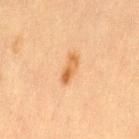- follow-up: no biopsy performed (imaged during a skin exam)
- image source: 15 mm crop, total-body photography
- site: the left thigh
- size: ~3.5 mm (longest diameter)
- patient: female, in their 50s
- illumination: cross-polarized